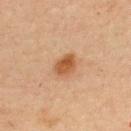Q: Is there a histopathology result?
A: total-body-photography surveillance lesion; no biopsy
Q: What lighting was used for the tile?
A: cross-polarized
Q: How was this image acquired?
A: ~15 mm tile from a whole-body skin photo
Q: What is the anatomic site?
A: the upper back
Q: What are the patient's age and sex?
A: female, roughly 45 years of age